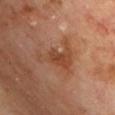{
  "patient": {
    "sex": "male",
    "age_approx": 70
  },
  "lighting": "cross-polarized",
  "site": "chest",
  "lesion_size": {
    "long_diameter_mm_approx": 5.5
  },
  "image": {
    "source": "total-body photography crop",
    "field_of_view_mm": 15
  }
}